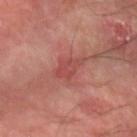Findings:
• follow-up · no biopsy performed (imaged during a skin exam)